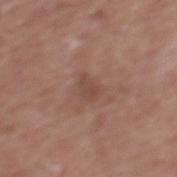Clinical impression: No biopsy was performed on this lesion — it was imaged during a full skin examination and was not determined to be concerning. Background: Automated tile analysis of the lesion measured an area of roughly 3 mm², a shape eccentricity near 0.8, and two-axis asymmetry of about 0.3. The software also gave a color-variation rating of about 1.5/10 and a peripheral color-asymmetry measure near 0.5. The lesion is located on the mid back. A male patient approximately 75 years of age. Approximately 2.5 mm at its widest. This image is a 15 mm lesion crop taken from a total-body photograph. The tile uses white-light illumination.